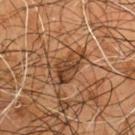Clinical impression: Captured during whole-body skin photography for melanoma surveillance; the lesion was not biopsied. Clinical summary: The total-body-photography lesion software estimated a mean CIELAB color near L≈39 a*≈19 b*≈31, a lesion–skin lightness drop of about 10, and a lesion-to-skin contrast of about 8 (normalized; higher = more distinct). Cropped from a whole-body photographic skin survey; the tile spans about 15 mm. The tile uses cross-polarized illumination. On the chest. The recorded lesion diameter is about 5 mm. A male subject, in their mid- to late 50s.